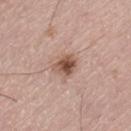notes = imaged on a skin check; not biopsied | image = total-body-photography crop, ~15 mm field of view | anatomic site = the left thigh | illumination = white-light | subject = male, approximately 55 years of age | lesion diameter = about 3 mm.